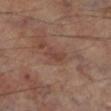Assessment: This lesion was catalogued during total-body skin photography and was not selected for biopsy. Acquisition and patient details: From the left lower leg. A 15 mm close-up extracted from a 3D total-body photography capture. About 2.5 mm across. This is a cross-polarized tile. A male patient, aged around 70.